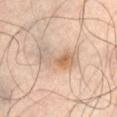| key | value |
|---|---|
| workup | imaged on a skin check; not biopsied |
| illumination | cross-polarized |
| image | total-body-photography crop, ~15 mm field of view |
| anatomic site | the abdomen |
| patient | male, aged 63–67 |
| size | ≈5.5 mm |